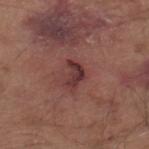Imaged during a routine full-body skin examination; the lesion was not biopsied and no histopathology is available.
A male patient approximately 80 years of age.
A region of skin cropped from a whole-body photographic capture, roughly 15 mm wide.
About 3.5 mm across.
The lesion is on the right lower leg.